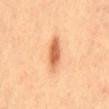workup: imaged on a skin check; not biopsied | lesion size: ~5 mm (longest diameter) | location: the mid back | tile lighting: cross-polarized illumination | acquisition: total-body-photography crop, ~15 mm field of view | patient: male, about 40 years old.